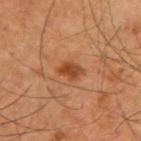The lesion was tiled from a total-body skin photograph and was not biopsied. The patient is a male about 60 years old. Measured at roughly 2.5 mm in maximum diameter. Located on the upper back. Imaged with cross-polarized lighting. A 15 mm close-up tile from a total-body photography series done for melanoma screening.